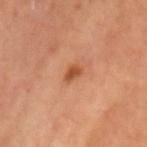This lesion was catalogued during total-body skin photography and was not selected for biopsy.
A 15 mm close-up tile from a total-body photography series done for melanoma screening.
Imaged with cross-polarized lighting.
From the left upper arm.
A female subject, aged approximately 70.
Longest diameter approximately 2 mm.